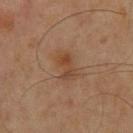The lesion is on the chest.
A male subject aged around 60.
Measured at roughly 3.5 mm in maximum diameter.
The lesion-visualizer software estimated a lesion area of about 4.5 mm², an eccentricity of roughly 0.9, and a shape-asymmetry score of about 0.35 (0 = symmetric). The software also gave an average lesion color of about L≈39 a*≈18 b*≈30 (CIELAB), roughly 7 lightness units darker than nearby skin, and a lesion-to-skin contrast of about 6.5 (normalized; higher = more distinct). The analysis additionally found an automated nevus-likeness rating near 20 out of 100 and a detector confidence of about 100 out of 100 that the crop contains a lesion.
This is a cross-polarized tile.
A 15 mm crop from a total-body photograph taken for skin-cancer surveillance.